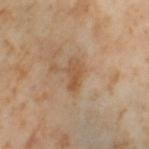Captured during whole-body skin photography for melanoma surveillance; the lesion was not biopsied. The subject is a female roughly 55 years of age. This image is a 15 mm lesion crop taken from a total-body photograph. Located on the left thigh.